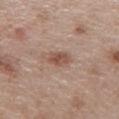The lesion's longest dimension is about 3 mm.
A roughly 15 mm field-of-view crop from a total-body skin photograph.
A female patient, roughly 40 years of age.
From the chest.
An algorithmic analysis of the crop reported a lesion area of about 5 mm², a shape eccentricity near 0.7, and a symmetry-axis asymmetry near 0.25. The software also gave a lesion color around L≈52 a*≈19 b*≈27 in CIELAB, roughly 10 lightness units darker than nearby skin, and a normalized border contrast of about 7. And it measured a nevus-likeness score of about 75/100 and lesion-presence confidence of about 100/100.